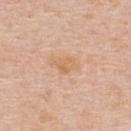• body site — the upper back
• automated metrics — an area of roughly 4 mm², an eccentricity of roughly 0.75, and a shape-asymmetry score of about 0.4 (0 = symmetric)
• imaging modality — ~15 mm crop, total-body skin-cancer survey
• tile lighting — white-light illumination
• subject — male, approximately 60 years of age
• diameter — ≈2.5 mm